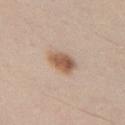{"biopsy_status": "not biopsied; imaged during a skin examination", "lesion_size": {"long_diameter_mm_approx": 3.5}, "image": {"source": "total-body photography crop", "field_of_view_mm": 15}, "site": "arm", "patient": {"sex": "male", "age_approx": 30}}